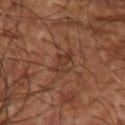Clinical impression: Captured during whole-body skin photography for melanoma surveillance; the lesion was not biopsied. Acquisition and patient details: A region of skin cropped from a whole-body photographic capture, roughly 15 mm wide. The recorded lesion diameter is about 3.5 mm. The total-body-photography lesion software estimated a lesion area of about 4 mm², a shape eccentricity near 0.9, and two-axis asymmetry of about 0.45. It also reported an automated nevus-likeness rating near 0 out of 100. Captured under cross-polarized illumination. On the right upper arm. The subject is a male roughly 60 years of age.